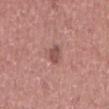Imaged during a routine full-body skin examination; the lesion was not biopsied and no histopathology is available.
A roughly 15 mm field-of-view crop from a total-body skin photograph.
The lesion is on the mid back.
A male subject, roughly 60 years of age.